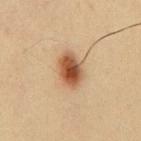Q: Was this lesion biopsied?
A: imaged on a skin check; not biopsied
Q: What lighting was used for the tile?
A: cross-polarized illumination
Q: What is the anatomic site?
A: the mid back
Q: Who is the patient?
A: male, in their mid- to late 30s
Q: Lesion size?
A: ~4.5 mm (longest diameter)
Q: What kind of image is this?
A: ~15 mm tile from a whole-body skin photo